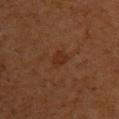Assessment: Part of a total-body skin-imaging series; this lesion was reviewed on a skin check and was not flagged for biopsy. Clinical summary: The lesion is on the upper back. The patient is a female approximately 50 years of age. The lesion-visualizer software estimated a lesion area of about 4 mm², an eccentricity of roughly 0.7, and a shape-asymmetry score of about 0.3 (0 = symmetric). It also reported border irregularity of about 2.5 on a 0–10 scale and a color-variation rating of about 2/10. And it measured a classifier nevus-likeness of about 0/100 and a detector confidence of about 100 out of 100 that the crop contains a lesion. A 15 mm close-up extracted from a 3D total-body photography capture. Imaged with cross-polarized lighting. The lesion's longest dimension is about 2.5 mm.